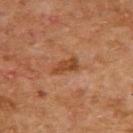notes: no biopsy performed (imaged during a skin exam) | image: total-body-photography crop, ~15 mm field of view | patient: female, about 60 years old | lesion size: about 3.5 mm | TBP lesion metrics: a lesion color around L≈40 a*≈22 b*≈32 in CIELAB, about 8 CIELAB-L* units darker than the surrounding skin, and a lesion-to-skin contrast of about 7.5 (normalized; higher = more distinct); a nevus-likeness score of about 0/100 and lesion-presence confidence of about 100/100 | illumination: cross-polarized illumination | anatomic site: the back.